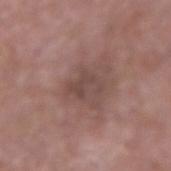<lesion>
  <biopsy_status>not biopsied; imaged during a skin examination</biopsy_status>
  <patient>
    <sex>male</sex>
    <age_approx>65</age_approx>
  </patient>
  <image>
    <source>total-body photography crop</source>
    <field_of_view_mm>15</field_of_view_mm>
  </image>
  <lighting>white-light</lighting>
  <automated_metrics>
    <area_mm2_approx>5.5</area_mm2_approx>
    <eccentricity>0.8</eccentricity>
    <shape_asymmetry>0.4</shape_asymmetry>
    <cielab_L>45</cielab_L>
    <cielab_a>18</cielab_a>
    <cielab_b>21</cielab_b>
    <vs_skin_darker_L>7.0</vs_skin_darker_L>
    <vs_skin_contrast_norm>6.0</vs_skin_contrast_norm>
    <nevus_likeness_0_100>0</nevus_likeness_0_100>
    <lesion_detection_confidence_0_100>95</lesion_detection_confidence_0_100>
  </automated_metrics>
  <site>left forearm</site>
  <lesion_size>
    <long_diameter_mm_approx>3.0</long_diameter_mm_approx>
  </lesion_size>
</lesion>